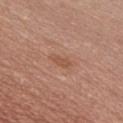<tbp_lesion>
<biopsy_status>not biopsied; imaged during a skin examination</biopsy_status>
<image>
  <source>total-body photography crop</source>
  <field_of_view_mm>15</field_of_view_mm>
</image>
<patient>
  <sex>male</sex>
  <age_approx>40</age_approx>
</patient>
<site>chest</site>
<lighting>white-light</lighting>
</tbp_lesion>